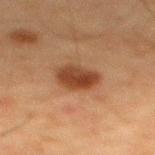The lesion was tiled from a total-body skin photograph and was not biopsied. This is a cross-polarized tile. A lesion tile, about 15 mm wide, cut from a 3D total-body photograph. Located on the mid back. The subject is a male roughly 60 years of age. Longest diameter approximately 4 mm.